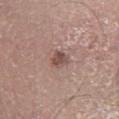Q: What lighting was used for the tile?
A: white-light illumination
Q: What are the patient's age and sex?
A: male, aged around 65
Q: Where on the body is the lesion?
A: the leg
Q: How was this image acquired?
A: 15 mm crop, total-body photography
Q: How large is the lesion?
A: ~2.5 mm (longest diameter)